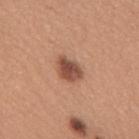Imaged during a routine full-body skin examination; the lesion was not biopsied and no histopathology is available.
A female patient, roughly 30 years of age.
On the upper back.
The recorded lesion diameter is about 3.5 mm.
Automated tile analysis of the lesion measured an area of roughly 6.5 mm² and an eccentricity of roughly 0.75.
A close-up tile cropped from a whole-body skin photograph, about 15 mm across.